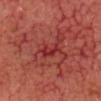biopsy status=imaged on a skin check; not biopsied | image-analysis metrics=a border-irregularity rating of about 4.5/10, internal color variation of about 2.5 on a 0–10 scale, and peripheral color asymmetry of about 1 | image=~15 mm tile from a whole-body skin photo | anatomic site=the head or neck | tile lighting=cross-polarized illumination | lesion diameter=about 3 mm | subject=aged 63 to 67.